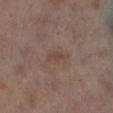<record>
<biopsy_status>not biopsied; imaged during a skin examination</biopsy_status>
<site>left lower leg</site>
<patient>
  <sex>male</sex>
  <age_approx>65</age_approx>
</patient>
<image>
  <source>total-body photography crop</source>
  <field_of_view_mm>15</field_of_view_mm>
</image>
<lesion_size>
  <long_diameter_mm_approx>2.5</long_diameter_mm_approx>
</lesion_size>
<lighting>cross-polarized</lighting>
</record>